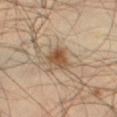Acquisition and patient details:
An algorithmic analysis of the crop reported a lesion area of about 6.5 mm² and a shape eccentricity near 0.4. It also reported a lesion color around L≈50 a*≈17 b*≈31 in CIELAB, roughly 11 lightness units darker than nearby skin, and a normalized lesion–skin contrast near 8.5. The analysis additionally found an automated nevus-likeness rating near 85 out of 100 and a lesion-detection confidence of about 100/100. Longest diameter approximately 3 mm. A 15 mm close-up tile from a total-body photography series done for melanoma screening. The lesion is on the left thigh. A male patient aged around 45.